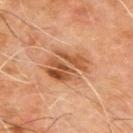biopsy status = catalogued during a skin exam; not biopsied
body site = the back
imaging modality = ~15 mm crop, total-body skin-cancer survey
patient = male, aged 63 to 67